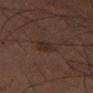biopsy_status: not biopsied; imaged during a skin examination
patient:
  sex: male
  age_approx: 60
automated_metrics:
  area_mm2_approx: 5.0
  eccentricity: 0.6
  shape_asymmetry: 0.25
  border_irregularity_0_10: 2.0
  color_variation_0_10: 1.5
  peripheral_color_asymmetry: 0.5
lighting: cross-polarized
lesion_size:
  long_diameter_mm_approx: 2.5
image:
  source: total-body photography crop
  field_of_view_mm: 15